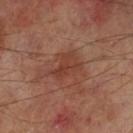follow-up = imaged on a skin check; not biopsied
acquisition = 15 mm crop, total-body photography
illumination = cross-polarized illumination
diameter = ~4 mm (longest diameter)
anatomic site = the left lower leg
subject = male, roughly 70 years of age